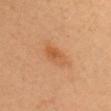Imaged during a routine full-body skin examination; the lesion was not biopsied and no histopathology is available.
A close-up tile cropped from a whole-body skin photograph, about 15 mm across.
Approximately 3.5 mm at its widest.
The tile uses cross-polarized illumination.
From the chest.
Automated image analysis of the tile measured a border-irregularity rating of about 2.5/10, internal color variation of about 5 on a 0–10 scale, and peripheral color asymmetry of about 1.5. The software also gave a classifier nevus-likeness of about 50/100 and a lesion-detection confidence of about 100/100.
A female patient aged around 40.